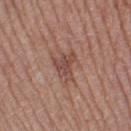The lesion was photographed on a routine skin check and not biopsied; there is no pathology result.
Located on the right thigh.
Automated tile analysis of the lesion measured an area of roughly 6 mm² and a shape-asymmetry score of about 0.45 (0 = symmetric). And it measured a lesion color around L≈47 a*≈21 b*≈24 in CIELAB and a normalized lesion–skin contrast near 7. The analysis additionally found internal color variation of about 3 on a 0–10 scale and peripheral color asymmetry of about 1.
This image is a 15 mm lesion crop taken from a total-body photograph.
About 3 mm across.
A female subject in their mid-50s.